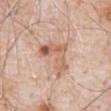Imaged during a routine full-body skin examination; the lesion was not biopsied and no histopathology is available.
The subject is a male in their 80s.
From the abdomen.
Captured under white-light illumination.
Measured at roughly 5.5 mm in maximum diameter.
A close-up tile cropped from a whole-body skin photograph, about 15 mm across.
The lesion-visualizer software estimated a border-irregularity rating of about 7.5/10, internal color variation of about 9.5 on a 0–10 scale, and a peripheral color-asymmetry measure near 4.5. The software also gave a classifier nevus-likeness of about 40/100 and lesion-presence confidence of about 100/100.